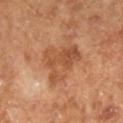Impression:
Captured during whole-body skin photography for melanoma surveillance; the lesion was not biopsied.
Context:
This image is a 15 mm lesion crop taken from a total-body photograph. Imaged with cross-polarized lighting. Measured at roughly 5.5 mm in maximum diameter. The lesion-visualizer software estimated a lesion color around L≈50 a*≈24 b*≈35 in CIELAB and a lesion-to-skin contrast of about 6.5 (normalized; higher = more distinct). The analysis additionally found a border-irregularity index near 8.5/10, a color-variation rating of about 4.5/10, and peripheral color asymmetry of about 1.5. The subject is a male aged around 65.